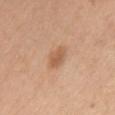biopsy_status: not biopsied; imaged during a skin examination
lesion_size:
  long_diameter_mm_approx: 3.0
image:
  source: total-body photography crop
  field_of_view_mm: 15
automated_metrics:
  cielab_L: 58
  cielab_a: 21
  cielab_b: 34
  vs_skin_darker_L: 10.0
  border_irregularity_0_10: 1.5
  color_variation_0_10: 1.5
  peripheral_color_asymmetry: 0.5
  nevus_likeness_0_100: 70
  lesion_detection_confidence_0_100: 100
site: chest
patient:
  sex: female
  age_approx: 30